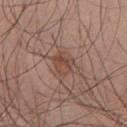biopsy_status: not biopsied; imaged during a skin examination
site: chest
patient:
  sex: male
  age_approx: 30
image:
  source: total-body photography crop
  field_of_view_mm: 15